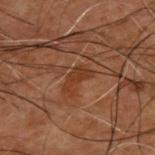No biopsy was performed on this lesion — it was imaged during a full skin examination and was not determined to be concerning.